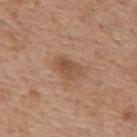Clinical summary:
The lesion is located on the mid back. A roughly 15 mm field-of-view crop from a total-body skin photograph. The lesion's longest dimension is about 3 mm. A male patient, in their 60s.